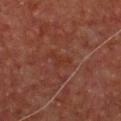Captured during whole-body skin photography for melanoma surveillance; the lesion was not biopsied.
The lesion is on the chest.
A male patient, approximately 65 years of age.
A region of skin cropped from a whole-body photographic capture, roughly 15 mm wide.
Longest diameter approximately 3 mm.
Captured under cross-polarized illumination.
Automated tile analysis of the lesion measured an average lesion color of about L≈30 a*≈23 b*≈26 (CIELAB) and roughly 4 lightness units darker than nearby skin.